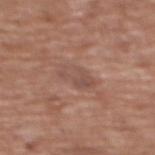No biopsy was performed on this lesion — it was imaged during a full skin examination and was not determined to be concerning. Imaged with white-light lighting. A female patient aged 73–77. The lesion's longest dimension is about 4 mm. The lesion is located on the upper back. A 15 mm close-up extracted from a 3D total-body photography capture.